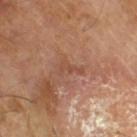Clinical impression: Captured during whole-body skin photography for melanoma surveillance; the lesion was not biopsied. Image and clinical context: The recorded lesion diameter is about 3 mm. A male patient in their mid- to late 60s. The lesion-visualizer software estimated a footprint of about 3.5 mm² and a symmetry-axis asymmetry near 0.55. It also reported a border-irregularity rating of about 6.5/10, a color-variation rating of about 0/10, and a peripheral color-asymmetry measure near 0. A 15 mm close-up tile from a total-body photography series done for melanoma screening.